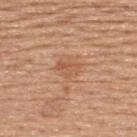workup — imaged on a skin check; not biopsied